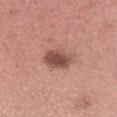A female subject, aged approximately 20. From the head or neck. The tile uses white-light illumination. The lesion-visualizer software estimated an automated nevus-likeness rating near 80 out of 100. Cropped from a whole-body photographic skin survey; the tile spans about 15 mm. Measured at roughly 3.5 mm in maximum diameter.